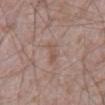| field | value |
|---|---|
| workup | total-body-photography surveillance lesion; no biopsy |
| subject | male, aged approximately 65 |
| size | ~3 mm (longest diameter) |
| site | the abdomen |
| imaging modality | ~15 mm tile from a whole-body skin photo |
| automated lesion analysis | a lesion area of about 3.5 mm², a shape eccentricity near 0.9, and two-axis asymmetry of about 0.3; an average lesion color of about L≈53 a*≈17 b*≈24 (CIELAB), a lesion–skin lightness drop of about 6, and a normalized border contrast of about 5; a within-lesion color-variation index near 1/10 and a peripheral color-asymmetry measure near 0; a nevus-likeness score of about 0/100 and lesion-presence confidence of about 100/100 |
| tile lighting | white-light illumination |